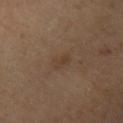{
  "biopsy_status": "not biopsied; imaged during a skin examination",
  "image": {
    "source": "total-body photography crop",
    "field_of_view_mm": 15
  },
  "site": "left upper arm",
  "lighting": "cross-polarized",
  "lesion_size": {
    "long_diameter_mm_approx": 2.5
  },
  "patient": {
    "sex": "female",
    "age_approx": 40
  }
}